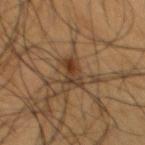Captured during whole-body skin photography for melanoma surveillance; the lesion was not biopsied.
The total-body-photography lesion software estimated a lesion color around L≈33 a*≈15 b*≈27 in CIELAB and a lesion-to-skin contrast of about 8.5 (normalized; higher = more distinct). And it measured border irregularity of about 3.5 on a 0–10 scale, internal color variation of about 7.5 on a 0–10 scale, and peripheral color asymmetry of about 2.5.
A male subject aged 53–57.
A close-up tile cropped from a whole-body skin photograph, about 15 mm across.
Imaged with cross-polarized lighting.
From the chest.